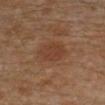biopsy status: catalogued during a skin exam; not biopsied
image: total-body-photography crop, ~15 mm field of view
patient: male, aged approximately 30
anatomic site: the left leg
automated lesion analysis: a footprint of about 8.5 mm², an outline eccentricity of about 0.55 (0 = round, 1 = elongated), and a shape-asymmetry score of about 0.2 (0 = symmetric); a mean CIELAB color near L≈38 a*≈20 b*≈29 and a normalized border contrast of about 5.5; a border-irregularity rating of about 2/10, a within-lesion color-variation index near 2/10, and radial color variation of about 1; a nevus-likeness score of about 15/100 and a lesion-detection confidence of about 100/100
tile lighting: cross-polarized
diameter: ~3.5 mm (longest diameter)